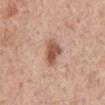Captured during whole-body skin photography for melanoma surveillance; the lesion was not biopsied.
An algorithmic analysis of the crop reported an outline eccentricity of about 0.75 (0 = round, 1 = elongated) and two-axis asymmetry of about 0.3. The software also gave a lesion–skin lightness drop of about 14 and a lesion-to-skin contrast of about 9 (normalized; higher = more distinct). And it measured border irregularity of about 2.5 on a 0–10 scale, a within-lesion color-variation index near 3.5/10, and radial color variation of about 1. It also reported an automated nevus-likeness rating near 90 out of 100.
The lesion is on the abdomen.
Approximately 3.5 mm at its widest.
Captured under white-light illumination.
A male subject, about 60 years old.
Cropped from a total-body skin-imaging series; the visible field is about 15 mm.